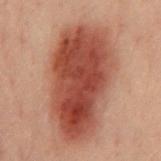biopsy status: total-body-photography surveillance lesion; no biopsy
image source: 15 mm crop, total-body photography
site: the chest
lesion diameter: about 11.5 mm
lighting: cross-polarized
patient: male, approximately 40 years of age
automated lesion analysis: an area of roughly 55 mm², an outline eccentricity of about 0.85 (0 = round, 1 = elongated), and a symmetry-axis asymmetry near 0.2; a mean CIELAB color near L≈37 a*≈23 b*≈25 and a normalized lesion–skin contrast near 11; a border-irregularity rating of about 2.5/10, a color-variation rating of about 6/10, and a peripheral color-asymmetry measure near 2; a classifier nevus-likeness of about 100/100